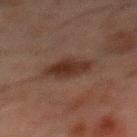<case>
<biopsy_status>not biopsied; imaged during a skin examination</biopsy_status>
<image>
  <source>total-body photography crop</source>
  <field_of_view_mm>15</field_of_view_mm>
</image>
<patient>
  <sex>male</sex>
  <age_approx>50</age_approx>
</patient>
<site>mid back</site>
<lighting>cross-polarized</lighting>
<lesion_size>
  <long_diameter_mm_approx>4.5</long_diameter_mm_approx>
</lesion_size>
<automated_metrics>
  <area_mm2_approx>9.5</area_mm2_approx>
  <shape_asymmetry>0.2</shape_asymmetry>
  <cielab_L>24</cielab_L>
  <cielab_a>15</cielab_a>
  <cielab_b>20</cielab_b>
  <vs_skin_contrast_norm>9.0</vs_skin_contrast_norm>
  <border_irregularity_0_10>2.5</border_irregularity_0_10>
  <color_variation_0_10>2.5</color_variation_0_10>
  <nevus_likeness_0_100>90</nevus_likeness_0_100>
  <lesion_detection_confidence_0_100>100</lesion_detection_confidence_0_100>
</automated_metrics>
</case>